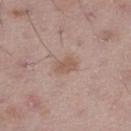biopsy status: total-body-photography surveillance lesion; no biopsy
tile lighting: white-light
automated metrics: a lesion area of about 4 mm², an eccentricity of roughly 0.8, and two-axis asymmetry of about 0.25; a border-irregularity index near 2.5/10, internal color variation of about 1.5 on a 0–10 scale, and peripheral color asymmetry of about 0.5
subject: male, about 50 years old
anatomic site: the left thigh
acquisition: ~15 mm tile from a whole-body skin photo
lesion diameter: ~3 mm (longest diameter)